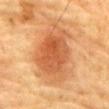Q: Was this lesion biopsied?
A: imaged on a skin check; not biopsied
Q: Who is the patient?
A: male, roughly 85 years of age or older
Q: What kind of image is this?
A: 15 mm crop, total-body photography
Q: What is the anatomic site?
A: the chest
Q: How was the tile lit?
A: cross-polarized
Q: Lesion size?
A: ≈8 mm
Q: Automated lesion metrics?
A: an average lesion color of about L≈51 a*≈24 b*≈37 (CIELAB), about 11 CIELAB-L* units darker than the surrounding skin, and a normalized lesion–skin contrast near 7.5